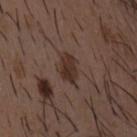Captured during whole-body skin photography for melanoma surveillance; the lesion was not biopsied.
Imaged with white-light lighting.
Automated image analysis of the tile measured a mean CIELAB color near L≈31 a*≈15 b*≈22 and a normalized lesion–skin contrast near 9. The software also gave a border-irregularity rating of about 2.5/10, a within-lesion color-variation index near 3/10, and radial color variation of about 1.
Measured at roughly 3.5 mm in maximum diameter.
From the chest.
Cropped from a total-body skin-imaging series; the visible field is about 15 mm.
A male subject aged 48–52.